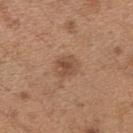Clinical summary:
The lesion-visualizer software estimated an area of roughly 5 mm² and a symmetry-axis asymmetry near 0.2. The analysis additionally found a classifier nevus-likeness of about 75/100 and a lesion-detection confidence of about 100/100. On the right upper arm. This is a white-light tile. Approximately 2.5 mm at its widest. A roughly 15 mm field-of-view crop from a total-body skin photograph. A female patient aged around 30.